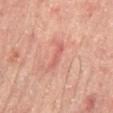Part of a total-body skin-imaging series; this lesion was reviewed on a skin check and was not flagged for biopsy. The lesion is located on the mid back. Longest diameter approximately 3.5 mm. The patient is a male aged 63–67. A close-up tile cropped from a whole-body skin photograph, about 15 mm across. Imaged with cross-polarized lighting.